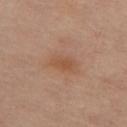| field | value |
|---|---|
| biopsy status | catalogued during a skin exam; not biopsied |